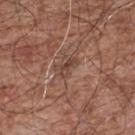Clinical impression:
No biopsy was performed on this lesion — it was imaged during a full skin examination and was not determined to be concerning.
Acquisition and patient details:
The total-body-photography lesion software estimated a lesion area of about 2.5 mm² and a shape-asymmetry score of about 0.35 (0 = symmetric). And it measured an average lesion color of about L≈42 a*≈21 b*≈25 (CIELAB), a lesion–skin lightness drop of about 9, and a lesion-to-skin contrast of about 7 (normalized; higher = more distinct). It also reported a border-irregularity index near 4/10. A male patient, about 55 years old. Captured under white-light illumination. Approximately 2.5 mm at its widest. Located on the upper back. A 15 mm close-up tile from a total-body photography series done for melanoma screening.